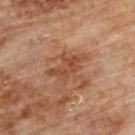Part of a total-body skin-imaging series; this lesion was reviewed on a skin check and was not flagged for biopsy. A male subject, roughly 85 years of age or older. Located on the upper back. A lesion tile, about 15 mm wide, cut from a 3D total-body photograph.